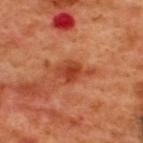{
  "biopsy_status": "not biopsied; imaged during a skin examination",
  "site": "back",
  "lighting": "cross-polarized",
  "image": {
    "source": "total-body photography crop",
    "field_of_view_mm": 15
  },
  "patient": {
    "sex": "male",
    "age_approx": 50
  }
}